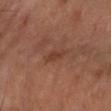  biopsy_status: not biopsied; imaged during a skin examination
  patient:
    sex: male
    age_approx: 55
  site: arm
  image:
    source: total-body photography crop
    field_of_view_mm: 15